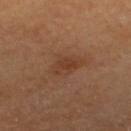notes: no biopsy performed (imaged during a skin exam) | TBP lesion metrics: a mean CIELAB color near L≈39 a*≈21 b*≈32 and a lesion-to-skin contrast of about 6 (normalized; higher = more distinct); border irregularity of about 4 on a 0–10 scale and a color-variation rating of about 1.5/10 | acquisition: ~15 mm tile from a whole-body skin photo | subject: female, aged 58–62 | body site: the left thigh | lighting: cross-polarized.